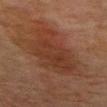Imaged during a routine full-body skin examination; the lesion was not biopsied and no histopathology is available. A 15 mm close-up extracted from a 3D total-body photography capture. A male patient, about 80 years old. Located on the front of the torso.